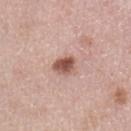The lesion is located on the left upper arm. This image is a 15 mm lesion crop taken from a total-body photograph. Imaged with white-light lighting. A female patient, about 50 years old.